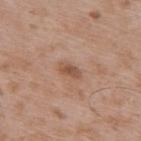| key | value |
|---|---|
| biopsy status | total-body-photography surveillance lesion; no biopsy |
| illumination | white-light |
| size | ~3 mm (longest diameter) |
| image source | total-body-photography crop, ~15 mm field of view |
| patient | male, aged 48 to 52 |
| location | the upper back |
| TBP lesion metrics | border irregularity of about 3 on a 0–10 scale, a color-variation rating of about 2/10, and a peripheral color-asymmetry measure near 0.5 |